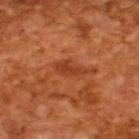follow-up: total-body-photography surveillance lesion; no biopsy
image: 15 mm crop, total-body photography
subject: male, approximately 65 years of age